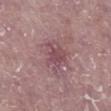Findings:
- follow-up — no biopsy performed (imaged during a skin exam)
- patient — male, aged 73 to 77
- body site — the right lower leg
- lesion diameter — ≈4 mm
- illumination — white-light
- image — ~15 mm crop, total-body skin-cancer survey